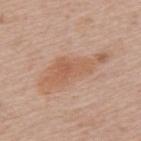Captured during whole-body skin photography for melanoma surveillance; the lesion was not biopsied. Cropped from a whole-body photographic skin survey; the tile spans about 15 mm. On the back. An algorithmic analysis of the crop reported a lesion–skin lightness drop of about 8 and a normalized border contrast of about 6.5. It also reported a classifier nevus-likeness of about 0/100. A female subject in their 60s. Approximately 8 mm at its widest.